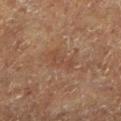Findings:
* workup: catalogued during a skin exam; not biopsied
* lesion size: ~4 mm (longest diameter)
* subject: male, aged 73–77
* image-analysis metrics: a footprint of about 4.5 mm², an eccentricity of roughly 0.95, and a shape-asymmetry score of about 0.5 (0 = symmetric); a nevus-likeness score of about 0/100
* image source: ~15 mm tile from a whole-body skin photo
* location: the leg
* illumination: cross-polarized illumination